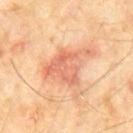- workup · catalogued during a skin exam; not biopsied
- patient · male, aged 58 to 62
- image · total-body-photography crop, ~15 mm field of view
- location · the chest